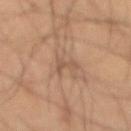Recorded during total-body skin imaging; not selected for excision or biopsy.
The total-body-photography lesion software estimated a shape eccentricity near 0.9 and a symmetry-axis asymmetry near 0.7. It also reported a border-irregularity index near 7/10, a color-variation rating of about 0/10, and a peripheral color-asymmetry measure near 0. The analysis additionally found a classifier nevus-likeness of about 0/100.
A roughly 15 mm field-of-view crop from a total-body skin photograph.
The lesion's longest dimension is about 3 mm.
This is a cross-polarized tile.
A male subject approximately 50 years of age.
On the back.